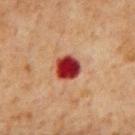site: mid back
image:
  source: total-body photography crop
  field_of_view_mm: 15
lesion_size:
  long_diameter_mm_approx: 3.0
patient:
  sex: male
  age_approx: 65